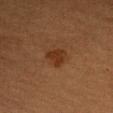No biopsy was performed on this lesion — it was imaged during a full skin examination and was not determined to be concerning.
The lesion is located on the arm.
The patient is a female in their mid-50s.
Cropped from a total-body skin-imaging series; the visible field is about 15 mm.
The recorded lesion diameter is about 2.5 mm.
Captured under cross-polarized illumination.